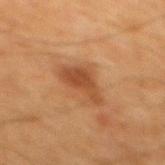Clinical impression:
The lesion was photographed on a routine skin check and not biopsied; there is no pathology result.
Acquisition and patient details:
The patient is a male about 65 years old. Automated image analysis of the tile measured a footprint of about 9.5 mm². And it measured a border-irregularity index near 4/10, internal color variation of about 2.5 on a 0–10 scale, and radial color variation of about 1. Measured at roughly 5 mm in maximum diameter. The tile uses cross-polarized illumination. Located on the mid back. A roughly 15 mm field-of-view crop from a total-body skin photograph.